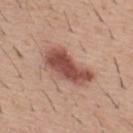Assessment:
No biopsy was performed on this lesion — it was imaged during a full skin examination and was not determined to be concerning.
Image and clinical context:
A roughly 15 mm field-of-view crop from a total-body skin photograph. The lesion is on the mid back. A male patient, about 40 years old.